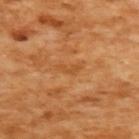<tbp_lesion>
<biopsy_status>not biopsied; imaged during a skin examination</biopsy_status>
<lighting>cross-polarized</lighting>
<image>
  <source>total-body photography crop</source>
  <field_of_view_mm>15</field_of_view_mm>
</image>
<patient>
  <sex>female</sex>
  <age_approx>55</age_approx>
</patient>
<automated_metrics>
  <area_mm2_approx>2.0</area_mm2_approx>
  <eccentricity>0.95</eccentricity>
  <shape_asymmetry>0.55</shape_asymmetry>
  <cielab_L>53</cielab_L>
  <cielab_a>26</cielab_a>
  <cielab_b>45</cielab_b>
  <vs_skin_contrast_norm>5.0</vs_skin_contrast_norm>
  <nevus_likeness_0_100>0</nevus_likeness_0_100>
  <lesion_detection_confidence_0_100>100</lesion_detection_confidence_0_100>
</automated_metrics>
<site>upper back</site>
</tbp_lesion>